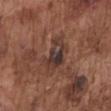The lesion was photographed on a routine skin check and not biopsied; there is no pathology result.
The recorded lesion diameter is about 4.5 mm.
The lesion is located on the chest.
A 15 mm close-up tile from a total-body photography series done for melanoma screening.
The subject is a male aged 73–77.